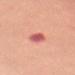Case summary:
• follow-up — catalogued during a skin exam; not biopsied
• site — the mid back
• TBP lesion metrics — a classifier nevus-likeness of about 5/100
• size — about 3 mm
• image — ~15 mm tile from a whole-body skin photo
• subject — female, aged 63–67
• lighting — white-light illumination